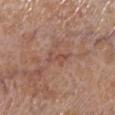The lesion was tiled from a total-body skin photograph and was not biopsied. The patient is a female approximately 55 years of age. A 15 mm crop from a total-body photograph taken for skin-cancer surveillance. The lesion-visualizer software estimated a footprint of about 3.5 mm², an eccentricity of roughly 0.9, and a symmetry-axis asymmetry near 0.4. The tile uses white-light illumination. From the left lower leg. The recorded lesion diameter is about 3 mm.